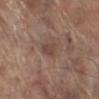{
  "biopsy_status": "not biopsied; imaged during a skin examination",
  "lighting": "white-light",
  "lesion_size": {
    "long_diameter_mm_approx": 2.5
  },
  "image": {
    "source": "total-body photography crop",
    "field_of_view_mm": 15
  },
  "site": "left lower leg",
  "patient": {
    "sex": "male",
    "age_approx": 70
  }
}